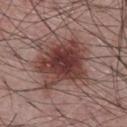Q: How large is the lesion?
A: ~6 mm (longest diameter)
Q: What are the patient's age and sex?
A: male, in their mid- to late 50s
Q: What is the imaging modality?
A: ~15 mm crop, total-body skin-cancer survey
Q: Illumination type?
A: white-light
Q: Lesion location?
A: the chest